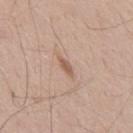Assessment:
The lesion was tiled from a total-body skin photograph and was not biopsied.
Context:
A roughly 15 mm field-of-view crop from a total-body skin photograph. A male patient about 55 years old.